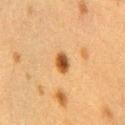Recorded during total-body skin imaging; not selected for excision or biopsy. The tile uses cross-polarized illumination. Approximately 2.5 mm at its widest. A female patient, about 40 years old. On the chest. A close-up tile cropped from a whole-body skin photograph, about 15 mm across. The total-body-photography lesion software estimated about 14 CIELAB-L* units darker than the surrounding skin and a lesion-to-skin contrast of about 10.5 (normalized; higher = more distinct).